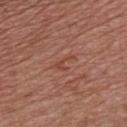notes: catalogued during a skin exam; not biopsied | size: ~3 mm (longest diameter) | body site: the chest | automated lesion analysis: an area of roughly 2.5 mm² and a shape eccentricity near 0.9; an average lesion color of about L≈45 a*≈25 b*≈30 (CIELAB), about 6 CIELAB-L* units darker than the surrounding skin, and a lesion-to-skin contrast of about 5.5 (normalized; higher = more distinct); a classifier nevus-likeness of about 0/100 and lesion-presence confidence of about 100/100 | patient: male, in their 70s | acquisition: 15 mm crop, total-body photography.